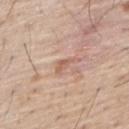No biopsy was performed on this lesion — it was imaged during a full skin examination and was not determined to be concerning.
A 15 mm close-up extracted from a 3D total-body photography capture.
From the back.
A male subject, aged 53–57.